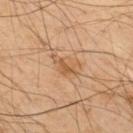The lesion was photographed on a routine skin check and not biopsied; there is no pathology result.
The lesion-visualizer software estimated an area of roughly 4.5 mm², an outline eccentricity of about 0.85 (0 = round, 1 = elongated), and two-axis asymmetry of about 0.45. And it measured border irregularity of about 7 on a 0–10 scale, a within-lesion color-variation index near 1.5/10, and a peripheral color-asymmetry measure near 0.5. It also reported a nevus-likeness score of about 5/100 and a detector confidence of about 100 out of 100 that the crop contains a lesion.
The tile uses cross-polarized illumination.
A male subject, roughly 50 years of age.
The recorded lesion diameter is about 4 mm.
A lesion tile, about 15 mm wide, cut from a 3D total-body photograph.
Located on the upper back.